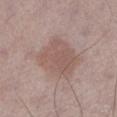Assessment: This lesion was catalogued during total-body skin photography and was not selected for biopsy. Clinical summary: The patient is a male approximately 60 years of age. An algorithmic analysis of the crop reported an average lesion color of about L≈55 a*≈17 b*≈22 (CIELAB), roughly 8 lightness units darker than nearby skin, and a normalized border contrast of about 6. On the left lower leg. The lesion's longest dimension is about 5 mm. A 15 mm crop from a total-body photograph taken for skin-cancer surveillance.